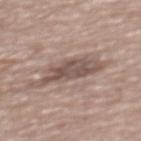Impression:
Recorded during total-body skin imaging; not selected for excision or biopsy.
Acquisition and patient details:
The lesion's longest dimension is about 7 mm. A lesion tile, about 15 mm wide, cut from a 3D total-body photograph. From the upper back. Automated tile analysis of the lesion measured a within-lesion color-variation index near 3.5/10 and peripheral color asymmetry of about 1. It also reported an automated nevus-likeness rating near 0 out of 100. Imaged with white-light lighting. A male subject aged approximately 80.